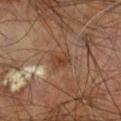This lesion was catalogued during total-body skin photography and was not selected for biopsy.
About 2.5 mm across.
An algorithmic analysis of the crop reported a lesion color around L≈40 a*≈20 b*≈29 in CIELAB, roughly 8 lightness units darker than nearby skin, and a normalized border contrast of about 7. The analysis additionally found a within-lesion color-variation index near 3/10.
On the right leg.
A male subject, aged approximately 60.
Captured under cross-polarized illumination.
A close-up tile cropped from a whole-body skin photograph, about 15 mm across.